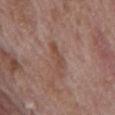| field | value |
|---|---|
| follow-up | imaged on a skin check; not biopsied |
| anatomic site | the mid back |
| imaging modality | ~15 mm tile from a whole-body skin photo |
| subject | male, in their 70s |
| lesion size | ≈3 mm |
| TBP lesion metrics | a lesion area of about 4 mm², an eccentricity of roughly 0.9, and a shape-asymmetry score of about 0.35 (0 = symmetric); a border-irregularity rating of about 4/10 and a within-lesion color-variation index near 1/10 |
| lighting | white-light illumination |